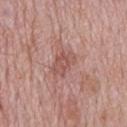{
  "biopsy_status": "not biopsied; imaged during a skin examination",
  "lesion_size": {
    "long_diameter_mm_approx": 3.5
  },
  "patient": {
    "sex": "male",
    "age_approx": 75
  },
  "image": {
    "source": "total-body photography crop",
    "field_of_view_mm": 15
  },
  "lighting": "white-light",
  "automated_metrics": {
    "area_mm2_approx": 5.5,
    "shape_asymmetry": 0.25
  },
  "site": "mid back"
}